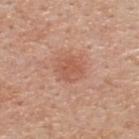Q: Was a biopsy performed?
A: no biopsy performed (imaged during a skin exam)
Q: What did automated image analysis measure?
A: a lesion area of about 6 mm², an outline eccentricity of about 0.35 (0 = round, 1 = elongated), and two-axis asymmetry of about 0.15; border irregularity of about 1.5 on a 0–10 scale, a within-lesion color-variation index near 2.5/10, and radial color variation of about 1
Q: Lesion location?
A: the upper back
Q: How large is the lesion?
A: about 3 mm
Q: Illumination type?
A: white-light illumination
Q: Patient demographics?
A: male, aged around 55
Q: What is the imaging modality?
A: 15 mm crop, total-body photography